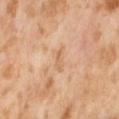| field | value |
|---|---|
| workup | imaged on a skin check; not biopsied |
| patient | female, in their mid-50s |
| illumination | cross-polarized |
| imaging modality | total-body-photography crop, ~15 mm field of view |
| location | the abdomen |